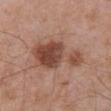From the abdomen. A 15 mm close-up extracted from a 3D total-body photography capture. Automated tile analysis of the lesion measured a lesion color around L≈47 a*≈22 b*≈27 in CIELAB, roughly 13 lightness units darker than nearby skin, and a normalized lesion–skin contrast near 9. The patient is a male aged approximately 70.